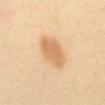This is a cross-polarized tile. A roughly 15 mm field-of-view crop from a total-body skin photograph. A female patient aged approximately 40. The lesion is on the abdomen. Measured at roughly 4.5 mm in maximum diameter.